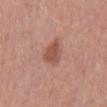The lesion is on the mid back.
Imaged with white-light lighting.
An algorithmic analysis of the crop reported a lesion area of about 6 mm², an outline eccentricity of about 0.65 (0 = round, 1 = elongated), and a symmetry-axis asymmetry near 0.25. The software also gave a mean CIELAB color near L≈52 a*≈25 b*≈28, roughly 10 lightness units darker than nearby skin, and a normalized border contrast of about 7.5. The software also gave border irregularity of about 2 on a 0–10 scale, a within-lesion color-variation index near 2.5/10, and peripheral color asymmetry of about 1.
A male patient aged around 70.
A roughly 15 mm field-of-view crop from a total-body skin photograph.